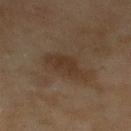Impression: Recorded during total-body skin imaging; not selected for excision or biopsy. Background: Located on the left lower leg. The subject is a female aged approximately 60. A lesion tile, about 15 mm wide, cut from a 3D total-body photograph.